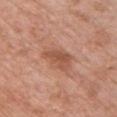Captured during whole-body skin photography for melanoma surveillance; the lesion was not biopsied. About 4 mm across. Automated image analysis of the tile measured a lesion area of about 9 mm², an eccentricity of roughly 0.8, and a shape-asymmetry score of about 0.25 (0 = symmetric). And it measured a border-irregularity index near 2.5/10, a within-lesion color-variation index near 3/10, and a peripheral color-asymmetry measure near 1. A 15 mm close-up extracted from a 3D total-body photography capture. This is a white-light tile. A female patient, approximately 50 years of age. The lesion is on the front of the torso.